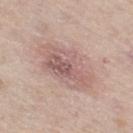Impression:
Imaged during a routine full-body skin examination; the lesion was not biopsied and no histopathology is available.
Acquisition and patient details:
The lesion's longest dimension is about 7 mm. A 15 mm close-up extracted from a 3D total-body photography capture. A female subject, roughly 65 years of age. Captured under white-light illumination. On the right thigh.